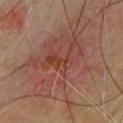Part of a total-body skin-imaging series; this lesion was reviewed on a skin check and was not flagged for biopsy. From the front of the torso. Cropped from a total-body skin-imaging series; the visible field is about 15 mm. An algorithmic analysis of the crop reported a border-irregularity rating of about 7.5/10 and peripheral color asymmetry of about 2. The analysis additionally found a classifier nevus-likeness of about 5/100 and lesion-presence confidence of about 100/100. A male patient about 45 years old. Measured at roughly 13.5 mm in maximum diameter.